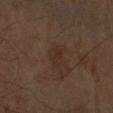Findings:
- biopsy status · imaged on a skin check; not biopsied
- location · the left arm
- image source · ~15 mm tile from a whole-body skin photo
- subject · male, roughly 65 years of age
- lesion size · ~2.5 mm (longest diameter)